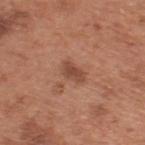Recorded during total-body skin imaging; not selected for excision or biopsy. Located on the upper back. This is a white-light tile. A male patient aged 63–67. Approximately 3 mm at its widest. Cropped from a total-body skin-imaging series; the visible field is about 15 mm. Automated image analysis of the tile measured a border-irregularity rating of about 2.5/10 and a peripheral color-asymmetry measure near 1. The analysis additionally found a classifier nevus-likeness of about 40/100.